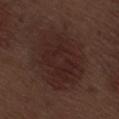Assessment: No biopsy was performed on this lesion — it was imaged during a full skin examination and was not determined to be concerning. Image and clinical context: The total-body-photography lesion software estimated border irregularity of about 2.5 on a 0–10 scale, a within-lesion color-variation index near 3/10, and radial color variation of about 1. The subject is a male aged 68–72. A 15 mm crop from a total-body photograph taken for skin-cancer surveillance. Located on the right thigh. The recorded lesion diameter is about 8 mm. Captured under white-light illumination.